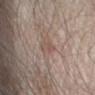No biopsy was performed on this lesion — it was imaged during a full skin examination and was not determined to be concerning. The lesion's longest dimension is about 2.5 mm. A male subject aged 78–82. Captured under white-light illumination. A 15 mm close-up tile from a total-body photography series done for melanoma screening. The lesion is located on the right forearm.